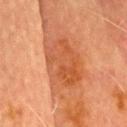This lesion was catalogued during total-body skin photography and was not selected for biopsy. The recorded lesion diameter is about 6.5 mm. A close-up tile cropped from a whole-body skin photograph, about 15 mm across. The total-body-photography lesion software estimated an area of roughly 24 mm², an outline eccentricity of about 0.75 (0 = round, 1 = elongated), and two-axis asymmetry of about 0.2. The software also gave a mean CIELAB color near L≈46 a*≈25 b*≈34 and about 8 CIELAB-L* units darker than the surrounding skin. The lesion is located on the head or neck. A male subject, aged around 70. Imaged with cross-polarized lighting.